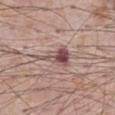Clinical impression:
No biopsy was performed on this lesion — it was imaged during a full skin examination and was not determined to be concerning.
Background:
A close-up tile cropped from a whole-body skin photograph, about 15 mm across. Automated tile analysis of the lesion measured a lesion area of about 7 mm² and a symmetry-axis asymmetry near 0.45. The analysis additionally found an average lesion color of about L≈51 a*≈19 b*≈20 (CIELAB), about 13 CIELAB-L* units darker than the surrounding skin, and a normalized border contrast of about 9. Captured under white-light illumination. Located on the abdomen. About 4.5 mm across. A male patient, roughly 70 years of age.